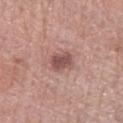Impression: Imaged during a routine full-body skin examination; the lesion was not biopsied and no histopathology is available. Background: An algorithmic analysis of the crop reported a lesion area of about 5.5 mm², an eccentricity of roughly 0.65, and a symmetry-axis asymmetry near 0.2. It also reported an average lesion color of about L≈51 a*≈22 b*≈22 (CIELAB) and a normalized lesion–skin contrast near 8. The software also gave a border-irregularity index near 2/10, internal color variation of about 2.5 on a 0–10 scale, and radial color variation of about 1. And it measured an automated nevus-likeness rating near 25 out of 100 and a detector confidence of about 100 out of 100 that the crop contains a lesion. The subject is a female aged 58–62. Captured under white-light illumination. The lesion is on the left forearm. The lesion's longest dimension is about 3 mm. A close-up tile cropped from a whole-body skin photograph, about 15 mm across.